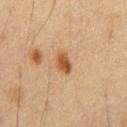follow-up: total-body-photography surveillance lesion; no biopsy | lighting: cross-polarized | imaging modality: 15 mm crop, total-body photography | lesion diameter: about 3 mm | patient: male, in their 60s | body site: the abdomen.